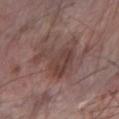biopsy_status: not biopsied; imaged during a skin examination
lighting: white-light
image:
  source: total-body photography crop
  field_of_view_mm: 15
lesion_size:
  long_diameter_mm_approx: 5.5
site: right forearm
automated_metrics:
  area_mm2_approx: 15.0
  eccentricity: 0.65
  shape_asymmetry: 0.55
  peripheral_color_asymmetry: 1.5
  lesion_detection_confidence_0_100: 95
patient:
  sex: male
  age_approx: 65